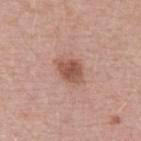Q: Was this lesion biopsied?
A: no biopsy performed (imaged during a skin exam)
Q: Automated lesion metrics?
A: a lesion area of about 6.5 mm² and two-axis asymmetry of about 0.25; about 12 CIELAB-L* units darker than the surrounding skin and a normalized lesion–skin contrast near 8.5; internal color variation of about 3.5 on a 0–10 scale and radial color variation of about 1; an automated nevus-likeness rating near 70 out of 100
Q: Lesion size?
A: ≈3.5 mm
Q: What lighting was used for the tile?
A: white-light illumination
Q: Patient demographics?
A: female, aged approximately 45
Q: How was this image acquired?
A: 15 mm crop, total-body photography
Q: What is the anatomic site?
A: the arm